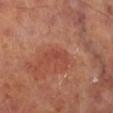The lesion was tiled from a total-body skin photograph and was not biopsied. The patient is a male aged 68 to 72. Located on the right lower leg. Cropped from a total-body skin-imaging series; the visible field is about 15 mm.